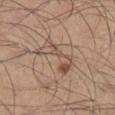Assessment: Recorded during total-body skin imaging; not selected for excision or biopsy. Acquisition and patient details: Measured at roughly 6 mm in maximum diameter. A 15 mm close-up extracted from a 3D total-body photography capture. A male subject aged 43 to 47. From the right lower leg.